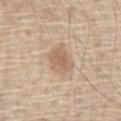<case>
<site>front of the torso</site>
<patient>
  <sex>male</sex>
  <age_approx>65</age_approx>
</patient>
<image>
  <source>total-body photography crop</source>
  <field_of_view_mm>15</field_of_view_mm>
</image>
</case>